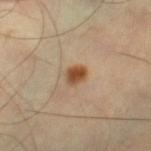biopsy status: catalogued during a skin exam; not biopsied
size: about 2.5 mm
anatomic site: the right thigh
tile lighting: cross-polarized illumination
patient: male, aged approximately 45
acquisition: total-body-photography crop, ~15 mm field of view